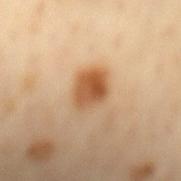follow-up = total-body-photography surveillance lesion; no biopsy
diameter = ≈3.5 mm
anatomic site = the mid back
illumination = cross-polarized illumination
subject = male, approximately 65 years of age
acquisition = ~15 mm tile from a whole-body skin photo